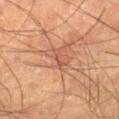workup — imaged on a skin check; not biopsied
image — total-body-photography crop, ~15 mm field of view
patient — male, approximately 65 years of age
illumination — cross-polarized illumination
automated metrics — a mean CIELAB color near L≈50 a*≈23 b*≈30 and a normalized border contrast of about 5; border irregularity of about 5.5 on a 0–10 scale, internal color variation of about 0 on a 0–10 scale, and peripheral color asymmetry of about 0; a nevus-likeness score of about 0/100 and a lesion-detection confidence of about 55/100
location — the left lower leg
diameter — ≈2.5 mm